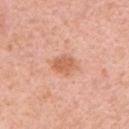Part of a total-body skin-imaging series; this lesion was reviewed on a skin check and was not flagged for biopsy. A male patient, about 60 years old. A 15 mm crop from a total-body photograph taken for skin-cancer surveillance. On the left upper arm.